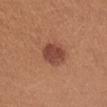Assessment:
The lesion was tiled from a total-body skin photograph and was not biopsied.
Clinical summary:
On the left forearm. A female patient, aged around 25. The tile uses white-light illumination. Measured at roughly 3.5 mm in maximum diameter. A 15 mm crop from a total-body photograph taken for skin-cancer surveillance.